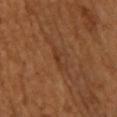Q: Was this lesion biopsied?
A: imaged on a skin check; not biopsied
Q: How was the tile lit?
A: cross-polarized illumination
Q: Lesion size?
A: ≈2.5 mm
Q: What kind of image is this?
A: 15 mm crop, total-body photography
Q: Patient demographics?
A: male, about 65 years old
Q: Where on the body is the lesion?
A: the upper back